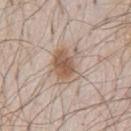Acquisition and patient details: A roughly 15 mm field-of-view crop from a total-body skin photograph. Imaged with white-light lighting. The lesion is on the chest. A male patient aged approximately 80. Approximately 4.5 mm at its widest.